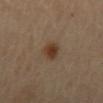Q: Was this lesion biopsied?
A: total-body-photography surveillance lesion; no biopsy
Q: What kind of image is this?
A: 15 mm crop, total-body photography
Q: Illumination type?
A: cross-polarized
Q: Patient demographics?
A: female, in their 60s
Q: Automated lesion metrics?
A: a lesion area of about 5 mm², an eccentricity of roughly 0.7, and a shape-asymmetry score of about 0.25 (0 = symmetric); a mean CIELAB color near L≈38 a*≈16 b*≈29, a lesion–skin lightness drop of about 9, and a normalized border contrast of about 9; a border-irregularity index near 2.5/10 and a within-lesion color-variation index near 4/10; a classifier nevus-likeness of about 95/100 and a lesion-detection confidence of about 100/100
Q: Where on the body is the lesion?
A: the lower back